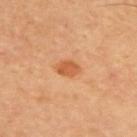Impression: No biopsy was performed on this lesion — it was imaged during a full skin examination and was not determined to be concerning. Acquisition and patient details: The lesion's longest dimension is about 2.5 mm. Imaged with cross-polarized lighting. The patient is a male aged around 65. Automated tile analysis of the lesion measured a footprint of about 4 mm² and a shape eccentricity near 0.75. The software also gave an average lesion color of about L≈58 a*≈28 b*≈42 (CIELAB) and a lesion-to-skin contrast of about 7.5 (normalized; higher = more distinct). And it measured a border-irregularity index near 1.5/10, internal color variation of about 2 on a 0–10 scale, and peripheral color asymmetry of about 1. On the upper back. A region of skin cropped from a whole-body photographic capture, roughly 15 mm wide.